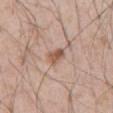Q: What is the anatomic site?
A: the abdomen
Q: Automated lesion metrics?
A: a border-irregularity rating of about 2.5/10, internal color variation of about 3.5 on a 0–10 scale, and radial color variation of about 1.5
Q: How large is the lesion?
A: about 3 mm
Q: What is the imaging modality?
A: total-body-photography crop, ~15 mm field of view
Q: What are the patient's age and sex?
A: male, aged 53 to 57